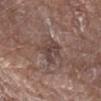follow-up: total-body-photography surveillance lesion; no biopsy | anatomic site: the arm | patient: male, aged approximately 80 | image: ~15 mm crop, total-body skin-cancer survey | diameter: ~4.5 mm (longest diameter) | illumination: white-light illumination.